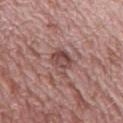{"biopsy_status": "not biopsied; imaged during a skin examination", "image": {"source": "total-body photography crop", "field_of_view_mm": 15}, "patient": {"sex": "male", "age_approx": 55}, "lighting": "white-light", "automated_metrics": {"area_mm2_approx": 6.5, "eccentricity": 0.55, "shape_asymmetry": 0.2, "border_irregularity_0_10": 2.0, "color_variation_0_10": 6.5, "peripheral_color_asymmetry": 2.0}, "site": "leg", "lesion_size": {"long_diameter_mm_approx": 3.0}}